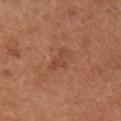Measured at roughly 3 mm in maximum diameter.
This is a white-light tile.
From the right upper arm.
Automated image analysis of the tile measured a lesion area of about 4.5 mm². The software also gave a lesion color around L≈46 a*≈24 b*≈33 in CIELAB, a lesion–skin lightness drop of about 6, and a normalized border contrast of about 5. The analysis additionally found a border-irregularity index near 5/10 and internal color variation of about 1 on a 0–10 scale. The analysis additionally found an automated nevus-likeness rating near 0 out of 100 and lesion-presence confidence of about 100/100.
This image is a 15 mm lesion crop taken from a total-body photograph.
The subject is a female aged approximately 65.